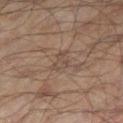Q: Was this lesion biopsied?
A: no biopsy performed (imaged during a skin exam)
Q: Lesion size?
A: ~3 mm (longest diameter)
Q: What are the patient's age and sex?
A: male, aged around 65
Q: What kind of image is this?
A: ~15 mm crop, total-body skin-cancer survey
Q: Automated lesion metrics?
A: an area of roughly 4 mm² and two-axis asymmetry of about 0.45; a lesion color around L≈44 a*≈15 b*≈23 in CIELAB, roughly 6 lightness units darker than nearby skin, and a normalized lesion–skin contrast near 5; a border-irregularity rating of about 4/10, a within-lesion color-variation index near 2.5/10, and a peripheral color-asymmetry measure near 1
Q: Where on the body is the lesion?
A: the right thigh
Q: What lighting was used for the tile?
A: cross-polarized